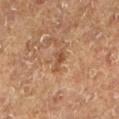Q: Was a biopsy performed?
A: no biopsy performed (imaged during a skin exam)
Q: Illumination type?
A: cross-polarized illumination
Q: What kind of image is this?
A: ~15 mm tile from a whole-body skin photo
Q: What are the patient's age and sex?
A: male, in their mid- to late 70s
Q: Where on the body is the lesion?
A: the left lower leg